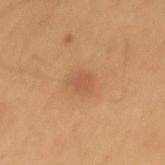body site: the mid back
illumination: cross-polarized
lesion diameter: ~2 mm (longest diameter)
subject: male, in their mid- to late 60s
image: total-body-photography crop, ~15 mm field of view
TBP lesion metrics: a border-irregularity rating of about 2.5/10, a within-lesion color-variation index near 0.5/10, and a peripheral color-asymmetry measure near 0; an automated nevus-likeness rating near 15 out of 100 and a lesion-detection confidence of about 100/100